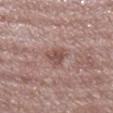Part of a total-body skin-imaging series; this lesion was reviewed on a skin check and was not flagged for biopsy. On the right lower leg. A 15 mm close-up tile from a total-body photography series done for melanoma screening. Approximately 2.5 mm at its widest. A male patient aged 73 to 77.